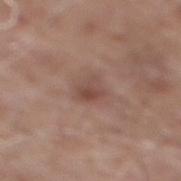notes=no biopsy performed (imaged during a skin exam) | patient=male, aged around 60 | acquisition=~15 mm crop, total-body skin-cancer survey | site=the mid back | lighting=white-light.